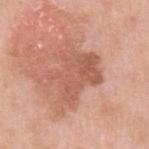biopsy status: no biopsy performed (imaged during a skin exam)
acquisition: 15 mm crop, total-body photography
automated lesion analysis: a lesion area of about 22 mm², a shape eccentricity near 0.65, and a symmetry-axis asymmetry near 0.45; border irregularity of about 7.5 on a 0–10 scale, internal color variation of about 4 on a 0–10 scale, and radial color variation of about 1.5; a classifier nevus-likeness of about 0/100
patient: male, approximately 60 years of age
illumination: white-light
location: the left upper arm